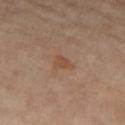Recorded during total-body skin imaging; not selected for excision or biopsy.
The lesion is on the right thigh.
The patient is a female roughly 75 years of age.
Cropped from a total-body skin-imaging series; the visible field is about 15 mm.
Longest diameter approximately 2.5 mm.
Imaged with cross-polarized lighting.